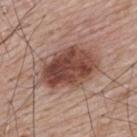follow-up: catalogued during a skin exam; not biopsied | automated lesion analysis: an average lesion color of about L≈46 a*≈21 b*≈25 (CIELAB) and a normalized border contrast of about 10.5; a border-irregularity index near 2.5/10 and a color-variation rating of about 6/10 | subject: male, about 65 years old | acquisition: 15 mm crop, total-body photography | lesion diameter: about 8 mm | body site: the upper back.